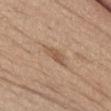Q: Is there a histopathology result?
A: catalogued during a skin exam; not biopsied
Q: Automated lesion metrics?
A: a border-irregularity rating of about 4/10, a color-variation rating of about 1.5/10, and radial color variation of about 0.5; an automated nevus-likeness rating near 0 out of 100 and a detector confidence of about 90 out of 100 that the crop contains a lesion
Q: Lesion size?
A: about 3.5 mm
Q: What are the patient's age and sex?
A: male, aged 68 to 72
Q: Lesion location?
A: the abdomen
Q: How was the tile lit?
A: white-light illumination
Q: What kind of image is this?
A: 15 mm crop, total-body photography